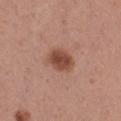anatomic site=the right thigh | image source=~15 mm tile from a whole-body skin photo | subject=female, aged approximately 30.